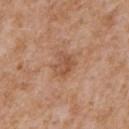Clinical impression:
Recorded during total-body skin imaging; not selected for excision or biopsy.
Acquisition and patient details:
The lesion is located on the chest. The tile uses white-light illumination. The patient is a male in their mid-60s. Measured at roughly 3 mm in maximum diameter. This image is a 15 mm lesion crop taken from a total-body photograph.